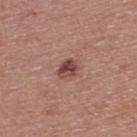Assessment: Captured during whole-body skin photography for melanoma surveillance; the lesion was not biopsied. Context: A male patient about 55 years old. Automated image analysis of the tile measured a shape eccentricity near 0.6 and a symmetry-axis asymmetry near 0.2. The software also gave a peripheral color-asymmetry measure near 1.5. It also reported an automated nevus-likeness rating near 70 out of 100 and a detector confidence of about 100 out of 100 that the crop contains a lesion. From the upper back. A region of skin cropped from a whole-body photographic capture, roughly 15 mm wide. The tile uses white-light illumination.